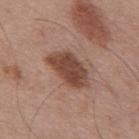Captured during whole-body skin photography for melanoma surveillance; the lesion was not biopsied. On the mid back. The subject is a male in their mid-50s. A 15 mm crop from a total-body photograph taken for skin-cancer surveillance. The tile uses white-light illumination.